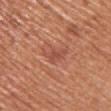workup: total-body-photography surveillance lesion; no biopsy | diameter: ~3 mm (longest diameter) | image source: ~15 mm tile from a whole-body skin photo | location: the chest | illumination: white-light | subject: female, about 60 years old | image-analysis metrics: an area of roughly 4 mm² and an eccentricity of roughly 0.8; an automated nevus-likeness rating near 5 out of 100.